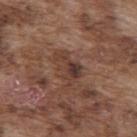* notes: no biopsy performed (imaged during a skin exam)
* lesion size: ~4.5 mm (longest diameter)
* location: the upper back
* subject: male, in their mid- to late 70s
* automated lesion analysis: a lesion-to-skin contrast of about 7 (normalized; higher = more distinct); an automated nevus-likeness rating near 0 out of 100 and a detector confidence of about 80 out of 100 that the crop contains a lesion
* tile lighting: white-light illumination
* acquisition: ~15 mm crop, total-body skin-cancer survey